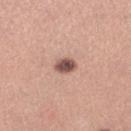{"biopsy_status": "not biopsied; imaged during a skin examination", "patient": {"sex": "female", "age_approx": 30}, "image": {"source": "total-body photography crop", "field_of_view_mm": 15}, "site": "right lower leg", "lighting": "white-light", "automated_metrics": {"area_mm2_approx": 4.5, "shape_asymmetry": 0.15, "vs_skin_contrast_norm": 11.0, "nevus_likeness_0_100": 90, "lesion_detection_confidence_0_100": 100}, "lesion_size": {"long_diameter_mm_approx": 3.0}}